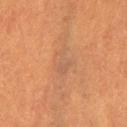Notes:
- biopsy status · imaged on a skin check; not biopsied
- site · the leg
- subject · female, aged 53 to 57
- tile lighting · cross-polarized
- automated metrics · an area of roughly 4.5 mm² and a shape-asymmetry score of about 0.5 (0 = symmetric); an average lesion color of about L≈48 a*≈18 b*≈30 (CIELAB), roughly 5 lightness units darker than nearby skin, and a normalized border contrast of about 4.5; border irregularity of about 6 on a 0–10 scale, internal color variation of about 1.5 on a 0–10 scale, and radial color variation of about 0.5; a nevus-likeness score of about 0/100
- diameter · about 4 mm
- acquisition · ~15 mm tile from a whole-body skin photo